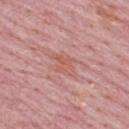The lesion was tiled from a total-body skin photograph and was not biopsied.
The tile uses white-light illumination.
A 15 mm crop from a total-body photograph taken for skin-cancer surveillance.
About 3.5 mm across.
A male subject aged approximately 65.
From the upper back.